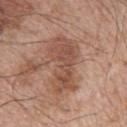No biopsy was performed on this lesion — it was imaged during a full skin examination and was not determined to be concerning.
Automated image analysis of the tile measured a lesion area of about 18 mm², an eccentricity of roughly 0.9, and a shape-asymmetry score of about 0.4 (0 = symmetric). It also reported internal color variation of about 3.5 on a 0–10 scale and a peripheral color-asymmetry measure near 1.
From the left upper arm.
A 15 mm close-up extracted from a 3D total-body photography capture.
Imaged with white-light lighting.
Approximately 7 mm at its widest.
The patient is a male approximately 65 years of age.